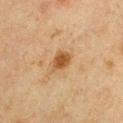illumination — cross-polarized illumination; subject — male, aged around 65; body site — the arm; diameter — ≈3 mm; acquisition — total-body-photography crop, ~15 mm field of view.